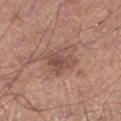• biopsy status — imaged on a skin check; not biopsied
• size — ≈5 mm
• patient — male, aged around 65
• image-analysis metrics — a footprint of about 12 mm² and an eccentricity of roughly 0.65; an average lesion color of about L≈50 a*≈20 b*≈25 (CIELAB), roughly 9 lightness units darker than nearby skin, and a normalized lesion–skin contrast near 6.5; a nevus-likeness score of about 5/100 and a lesion-detection confidence of about 100/100
• image — ~15 mm crop, total-body skin-cancer survey
• lighting — white-light illumination
• site — the left lower leg